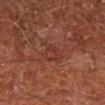notes: total-body-photography surveillance lesion; no biopsy | location: the right lower leg | image: ~15 mm crop, total-body skin-cancer survey | patient: male, roughly 65 years of age.